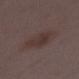{"biopsy_status": "not biopsied; imaged during a skin examination", "lighting": "white-light", "site": "right lower leg", "image": {"source": "total-body photography crop", "field_of_view_mm": 15}, "lesion_size": {"long_diameter_mm_approx": 4.5}, "patient": {"sex": "female", "age_approx": 30}, "automated_metrics": {"area_mm2_approx": 12.0, "shape_asymmetry": 0.3, "cielab_L": 34, "cielab_a": 14, "cielab_b": 17, "vs_skin_darker_L": 6.0, "border_irregularity_0_10": 3.0, "color_variation_0_10": 2.5, "nevus_likeness_0_100": 15, "lesion_detection_confidence_0_100": 100}}